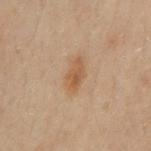The lesion was photographed on a routine skin check and not biopsied; there is no pathology result. From the chest. This image is a 15 mm lesion crop taken from a total-body photograph. Captured under cross-polarized illumination. Measured at roughly 4 mm in maximum diameter. A male subject, approximately 50 years of age.